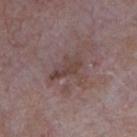The lesion was photographed on a routine skin check and not biopsied; there is no pathology result. Captured under white-light illumination. A region of skin cropped from a whole-body photographic capture, roughly 15 mm wide. A male patient, aged approximately 65. On the chest.